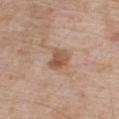Q: What are the patient's age and sex?
A: male, aged 63 to 67
Q: Lesion location?
A: the chest
Q: What kind of image is this?
A: total-body-photography crop, ~15 mm field of view
Q: What lighting was used for the tile?
A: white-light
Q: What did automated image analysis measure?
A: a footprint of about 6.5 mm², an outline eccentricity of about 0.5 (0 = round, 1 = elongated), and two-axis asymmetry of about 0.25; border irregularity of about 2.5 on a 0–10 scale and a peripheral color-asymmetry measure near 1.5
Q: Lesion size?
A: ~3 mm (longest diameter)